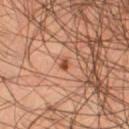notes = no biopsy performed (imaged during a skin exam)
lesion diameter = about 1.5 mm
TBP lesion metrics = a mean CIELAB color near L≈49 a*≈26 b*≈35 and a lesion–skin lightness drop of about 11; a classifier nevus-likeness of about 75/100 and a lesion-detection confidence of about 100/100
subject = male, aged approximately 45
site = the right thigh
image source = ~15 mm crop, total-body skin-cancer survey